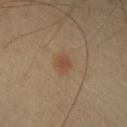The lesion was tiled from a total-body skin photograph and was not biopsied.
On the chest.
Imaged with cross-polarized lighting.
The lesion's longest dimension is about 1.5 mm.
A close-up tile cropped from a whole-body skin photograph, about 15 mm across.
A male patient, about 55 years old.
The total-body-photography lesion software estimated a lesion area of about 2.5 mm², an eccentricity of roughly 0.4, and two-axis asymmetry of about 0.25. And it measured a mean CIELAB color near L≈47 a*≈18 b*≈31, about 7 CIELAB-L* units darker than the surrounding skin, and a normalized border contrast of about 6.